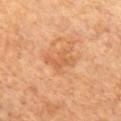This lesion was catalogued during total-body skin photography and was not selected for biopsy.